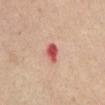This lesion was catalogued during total-body skin photography and was not selected for biopsy. A female patient aged approximately 65. Automated image analysis of the tile measured a mean CIELAB color near L≈57 a*≈35 b*≈28, about 15 CIELAB-L* units darker than the surrounding skin, and a normalized border contrast of about 9.5. The analysis additionally found a border-irregularity index near 2.5/10, internal color variation of about 5 on a 0–10 scale, and a peripheral color-asymmetry measure near 1.5. A region of skin cropped from a whole-body photographic capture, roughly 15 mm wide. The lesion is located on the abdomen. About 3 mm across. The tile uses white-light illumination.